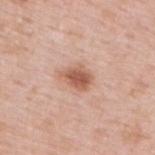Q: Is there a histopathology result?
A: imaged on a skin check; not biopsied
Q: What is the lesion's diameter?
A: ≈3.5 mm
Q: Patient demographics?
A: male, approximately 50 years of age
Q: What kind of image is this?
A: ~15 mm crop, total-body skin-cancer survey
Q: Where on the body is the lesion?
A: the upper back